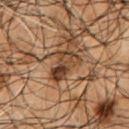The lesion was tiled from a total-body skin photograph and was not biopsied.
Measured at roughly 4 mm in maximum diameter.
Cropped from a whole-body photographic skin survey; the tile spans about 15 mm.
Captured under cross-polarized illumination.
On the chest.
A male subject aged 53 to 57.